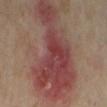This lesion was catalogued during total-body skin photography and was not selected for biopsy. This is a cross-polarized tile. Located on the right lower leg. About 13.5 mm across. An algorithmic analysis of the crop reported a border-irregularity index near 7/10 and a color-variation rating of about 7/10. A 15 mm close-up tile from a total-body photography series done for melanoma screening. A female subject, roughly 35 years of age.